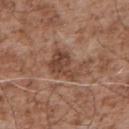Captured under white-light illumination. An algorithmic analysis of the crop reported an average lesion color of about L≈44 a*≈20 b*≈28 (CIELAB) and a lesion–skin lightness drop of about 10. And it measured a border-irregularity rating of about 6.5/10, a color-variation rating of about 4/10, and a peripheral color-asymmetry measure near 1.5. The analysis additionally found a nevus-likeness score of about 0/100 and a detector confidence of about 100 out of 100 that the crop contains a lesion. A male patient, aged around 55. A close-up tile cropped from a whole-body skin photograph, about 15 mm across. The lesion is located on the upper back.